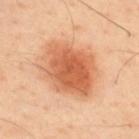  patient:
    sex: male
    age_approx: 45
  site: upper back
  image:
    source: total-body photography crop
    field_of_view_mm: 15